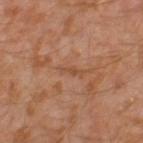notes — total-body-photography surveillance lesion; no biopsy | imaging modality — total-body-photography crop, ~15 mm field of view | location — the left thigh | diameter — about 3 mm | subject — male, aged approximately 30.